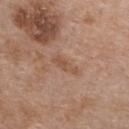Impression: The lesion was photographed on a routine skin check and not biopsied; there is no pathology result. Context: A region of skin cropped from a whole-body photographic capture, roughly 15 mm wide. The recorded lesion diameter is about 3.5 mm. The lesion is on the front of the torso. A male subject, aged approximately 65. This is a white-light tile.